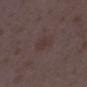Notes:
* size — about 3 mm
* TBP lesion metrics — a lesion area of about 5.5 mm², an eccentricity of roughly 0.65, and a shape-asymmetry score of about 0.2 (0 = symmetric); an average lesion color of about L≈34 a*≈14 b*≈17 (CIELAB) and a lesion-to-skin contrast of about 5 (normalized; higher = more distinct); a detector confidence of about 100 out of 100 that the crop contains a lesion
* image — 15 mm crop, total-body photography
* tile lighting — white-light illumination
* body site — the right lower leg
* subject — female, in their mid- to late 30s